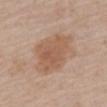Recorded during total-body skin imaging; not selected for excision or biopsy. A lesion tile, about 15 mm wide, cut from a 3D total-body photograph. The subject is a female aged 63–67. Imaged with white-light lighting. About 8 mm across. From the mid back.